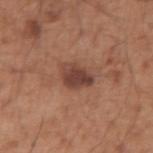Captured during whole-body skin photography for melanoma surveillance; the lesion was not biopsied. The lesion is on the right upper arm. Automated tile analysis of the lesion measured a shape eccentricity near 0.75 and two-axis asymmetry of about 0.3. The analysis additionally found a nevus-likeness score of about 75/100 and lesion-presence confidence of about 100/100. A 15 mm close-up tile from a total-body photography series done for melanoma screening. The patient is a male about 55 years old. This is a white-light tile. Longest diameter approximately 4 mm.